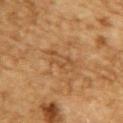Findings:
* workup: catalogued during a skin exam; not biopsied
* anatomic site: the upper back
* subject: male, in their mid-80s or older
* lighting: cross-polarized
* lesion size: about 4 mm
* image source: total-body-photography crop, ~15 mm field of view